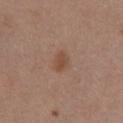follow-up: no biopsy performed (imaged during a skin exam) | image source: 15 mm crop, total-body photography | diameter: ~2.5 mm (longest diameter) | anatomic site: the chest | subject: female, aged around 55 | TBP lesion metrics: an outline eccentricity of about 0.75 (0 = round, 1 = elongated) and a symmetry-axis asymmetry near 0.2; a lesion color around L≈48 a*≈19 b*≈29 in CIELAB, roughly 7 lightness units darker than nearby skin, and a lesion-to-skin contrast of about 6.5 (normalized; higher = more distinct); a nevus-likeness score of about 55/100 and lesion-presence confidence of about 100/100.